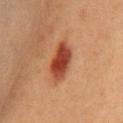Captured during whole-body skin photography for melanoma surveillance; the lesion was not biopsied.
This image is a 15 mm lesion crop taken from a total-body photograph.
Automated image analysis of the tile measured a mean CIELAB color near L≈38 a*≈28 b*≈31 and a lesion-to-skin contrast of about 11 (normalized; higher = more distinct). It also reported a border-irregularity rating of about 3/10 and a within-lesion color-variation index near 4/10. And it measured a classifier nevus-likeness of about 100/100.
Approximately 5 mm at its widest.
A female patient, in their 40s.
Located on the front of the torso.